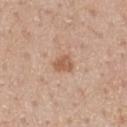Assessment: Captured during whole-body skin photography for melanoma surveillance; the lesion was not biopsied. Image and clinical context: Located on the front of the torso. A male subject, aged 53 to 57. This image is a 15 mm lesion crop taken from a total-body photograph.